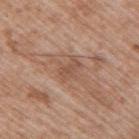No biopsy was performed on this lesion — it was imaged during a full skin examination and was not determined to be concerning. An algorithmic analysis of the crop reported an average lesion color of about L≈52 a*≈20 b*≈30 (CIELAB). The analysis additionally found a classifier nevus-likeness of about 0/100 and a detector confidence of about 100 out of 100 that the crop contains a lesion. A close-up tile cropped from a whole-body skin photograph, about 15 mm across. Captured under white-light illumination. A male subject, aged 48–52. The lesion is on the arm.